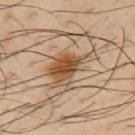{"biopsy_status": "not biopsied; imaged during a skin examination", "patient": {"sex": "male", "age_approx": 40}, "site": "left upper arm", "lighting": "cross-polarized", "image": {"source": "total-body photography crop", "field_of_view_mm": 15}}